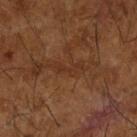Assessment:
The lesion was photographed on a routine skin check and not biopsied; there is no pathology result.
Clinical summary:
Automated image analysis of the tile measured a symmetry-axis asymmetry near 0.5. The analysis additionally found a color-variation rating of about 0/10 and radial color variation of about 0. And it measured an automated nevus-likeness rating near 0 out of 100 and a detector confidence of about 60 out of 100 that the crop contains a lesion. From the right forearm. Measured at roughly 3.5 mm in maximum diameter. The tile uses cross-polarized illumination. Cropped from a whole-body photographic skin survey; the tile spans about 15 mm. A male subject, aged 63 to 67.